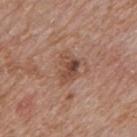Part of a total-body skin-imaging series; this lesion was reviewed on a skin check and was not flagged for biopsy. The lesion is on the mid back. A male subject, roughly 60 years of age. A 15 mm close-up tile from a total-body photography series done for melanoma screening.